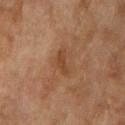Acquisition and patient details: A roughly 15 mm field-of-view crop from a total-body skin photograph. From the right upper arm. The lesion's longest dimension is about 3 mm. A female patient about 60 years old.